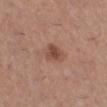Assessment: The lesion was tiled from a total-body skin photograph and was not biopsied. Background: An algorithmic analysis of the crop reported an area of roughly 5 mm², an eccentricity of roughly 0.7, and two-axis asymmetry of about 0.2. The analysis additionally found roughly 10 lightness units darker than nearby skin and a normalized lesion–skin contrast near 7.5. And it measured a border-irregularity index near 2/10 and a color-variation rating of about 3/10. A female patient, in their mid-40s. This is a white-light tile. From the right lower leg. A 15 mm crop from a total-body photograph taken for skin-cancer surveillance. The lesion's longest dimension is about 3 mm.